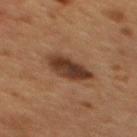Q: Was this lesion biopsied?
A: total-body-photography surveillance lesion; no biopsy
Q: What kind of image is this?
A: ~15 mm crop, total-body skin-cancer survey
Q: Patient demographics?
A: male, aged 53–57
Q: Where on the body is the lesion?
A: the mid back